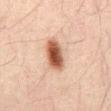Q: Was a biopsy performed?
A: catalogued during a skin exam; not biopsied
Q: What lighting was used for the tile?
A: cross-polarized illumination
Q: Where on the body is the lesion?
A: the front of the torso
Q: How was this image acquired?
A: total-body-photography crop, ~15 mm field of view
Q: How large is the lesion?
A: ≈4 mm
Q: Patient demographics?
A: male, in their 30s